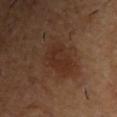Notes:
• notes: catalogued during a skin exam; not biopsied
• automated lesion analysis: a footprint of about 10 mm², a shape eccentricity near 0.85, and a symmetry-axis asymmetry near 0.25; border irregularity of about 2.5 on a 0–10 scale, a within-lesion color-variation index near 2.5/10, and radial color variation of about 1
• body site: the chest
• image: total-body-photography crop, ~15 mm field of view
• subject: male, roughly 55 years of age
• illumination: cross-polarized